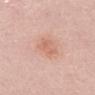Clinical impression:
The lesion was photographed on a routine skin check and not biopsied; there is no pathology result.
Image and clinical context:
A lesion tile, about 15 mm wide, cut from a 3D total-body photograph. Imaged with white-light lighting. On the mid back. Approximately 3 mm at its widest. The subject is a female aged around 50.